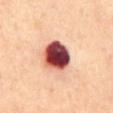Clinical impression: The lesion was tiled from a total-body skin photograph and was not biopsied. Background: The lesion-visualizer software estimated a shape eccentricity near 0.25 and a symmetry-axis asymmetry near 0.15. Captured under cross-polarized illumination. Cropped from a whole-body photographic skin survey; the tile spans about 15 mm. The lesion is on the abdomen. A male patient about 70 years old. The lesion's longest dimension is about 4 mm.